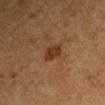| key | value |
|---|---|
| workup | no biopsy performed (imaged during a skin exam) |
| image | ~15 mm tile from a whole-body skin photo |
| subject | male, aged 63 to 67 |
| body site | the left upper arm |